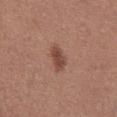{"biopsy_status": "not biopsied; imaged during a skin examination", "lesion_size": {"long_diameter_mm_approx": 3.5}, "automated_metrics": {"border_irregularity_0_10": 3.0, "color_variation_0_10": 3.0, "peripheral_color_asymmetry": 1.0}, "site": "chest", "patient": {"sex": "female", "age_approx": 50}, "image": {"source": "total-body photography crop", "field_of_view_mm": 15}}